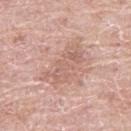Impression: The lesion was photographed on a routine skin check and not biopsied; there is no pathology result. Image and clinical context: A female patient aged around 60. Cropped from a total-body skin-imaging series; the visible field is about 15 mm. On the right thigh. Automated image analysis of the tile measured a lesion color around L≈61 a*≈21 b*≈26 in CIELAB, about 7 CIELAB-L* units darker than the surrounding skin, and a normalized border contrast of about 5. The analysis additionally found internal color variation of about 3 on a 0–10 scale and radial color variation of about 1. The software also gave an automated nevus-likeness rating near 0 out of 100 and a detector confidence of about 95 out of 100 that the crop contains a lesion.